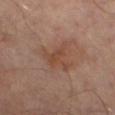biopsy status: no biopsy performed (imaged during a skin exam) | tile lighting: cross-polarized illumination | patient: male, approximately 60 years of age | anatomic site: the right leg | image source: ~15 mm tile from a whole-body skin photo | automated lesion analysis: a footprint of about 6.5 mm², an outline eccentricity of about 0.3 (0 = round, 1 = elongated), and a shape-asymmetry score of about 0.6 (0 = symmetric); a lesion color around L≈42 a*≈20 b*≈27 in CIELAB.